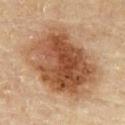workup = no biopsy performed (imaged during a skin exam)
subject = male, in their mid-80s or older
image = ~15 mm tile from a whole-body skin photo
image-analysis metrics = a lesion area of about 50 mm² and two-axis asymmetry of about 0.15; about 15 CIELAB-L* units darker than the surrounding skin
location = the chest
illumination = cross-polarized illumination
size = ≈9 mm